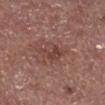workup — total-body-photography surveillance lesion; no biopsy
TBP lesion metrics — an area of roughly 7 mm² and an outline eccentricity of about 0.85 (0 = round, 1 = elongated); a border-irregularity rating of about 3.5/10, a within-lesion color-variation index near 4.5/10, and a peripheral color-asymmetry measure near 1.5
subject — male, in their mid- to late 50s
imaging modality — ~15 mm tile from a whole-body skin photo
diameter — ~4.5 mm (longest diameter)
illumination — white-light illumination
site — the leg